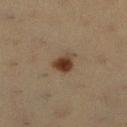The lesion was tiled from a total-body skin photograph and was not biopsied. The lesion-visualizer software estimated a border-irregularity index near 2.5/10 and peripheral color asymmetry of about 1. The software also gave an automated nevus-likeness rating near 100 out of 100 and a lesion-detection confidence of about 100/100. Captured under cross-polarized illumination. A female patient, aged 38 to 42. A region of skin cropped from a whole-body photographic capture, roughly 15 mm wide. The lesion is located on the right lower leg. Longest diameter approximately 3 mm.